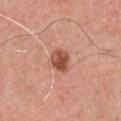The lesion was tiled from a total-body skin photograph and was not biopsied.
This image is a 15 mm lesion crop taken from a total-body photograph.
On the chest.
A male subject, approximately 60 years of age.
Captured under white-light illumination.